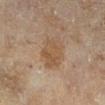Assessment:
The lesion was tiled from a total-body skin photograph and was not biopsied.
Context:
The lesion is located on the leg. Longest diameter approximately 4.5 mm. A region of skin cropped from a whole-body photographic capture, roughly 15 mm wide. A female subject in their 60s. The total-body-photography lesion software estimated an average lesion color of about L≈47 a*≈15 b*≈29 (CIELAB), a lesion–skin lightness drop of about 6, and a normalized border contrast of about 6. The analysis additionally found an automated nevus-likeness rating near 0 out of 100 and a lesion-detection confidence of about 100/100. Imaged with cross-polarized lighting.